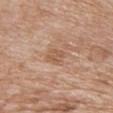Imaged during a routine full-body skin examination; the lesion was not biopsied and no histopathology is available. Located on the chest. The subject is a female aged approximately 70. The total-body-photography lesion software estimated a border-irregularity rating of about 3/10 and a color-variation rating of about 1.5/10. It also reported an automated nevus-likeness rating near 0 out of 100 and a detector confidence of about 100 out of 100 that the crop contains a lesion. About 2.5 mm across. A roughly 15 mm field-of-view crop from a total-body skin photograph. The tile uses white-light illumination.